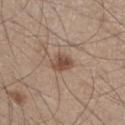No biopsy was performed on this lesion — it was imaged during a full skin examination and was not determined to be concerning.